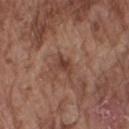Impression: Imaged during a routine full-body skin examination; the lesion was not biopsied and no histopathology is available. Acquisition and patient details: The patient is a male aged 73–77. From the right upper arm. Approximately 2.5 mm at its widest. A roughly 15 mm field-of-view crop from a total-body skin photograph. The total-body-photography lesion software estimated a mean CIELAB color near L≈40 a*≈21 b*≈26 and roughly 9 lightness units darker than nearby skin. And it measured a border-irregularity index near 3.5/10, internal color variation of about 2.5 on a 0–10 scale, and radial color variation of about 1.